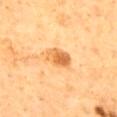body site = the back; subject = male, aged approximately 60; acquisition = ~15 mm tile from a whole-body skin photo; lighting = cross-polarized illumination.